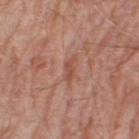This lesion was catalogued during total-body skin photography and was not selected for biopsy. A lesion tile, about 15 mm wide, cut from a 3D total-body photograph. This is a white-light tile. The subject is a male aged approximately 80. From the right thigh.